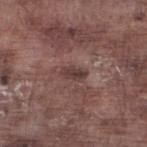Part of a total-body skin-imaging series; this lesion was reviewed on a skin check and was not flagged for biopsy. The lesion-visualizer software estimated an area of roughly 3.5 mm², an eccentricity of roughly 0.85, and a shape-asymmetry score of about 0.3 (0 = symmetric). The software also gave an automated nevus-likeness rating near 0 out of 100 and a lesion-detection confidence of about 90/100. Longest diameter approximately 3 mm. The tile uses white-light illumination. This image is a 15 mm lesion crop taken from a total-body photograph. Located on the left lower leg. A male patient, roughly 75 years of age.